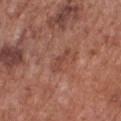notes: catalogued during a skin exam; not biopsied
location: the chest
illumination: white-light
image: total-body-photography crop, ~15 mm field of view
subject: male, roughly 75 years of age
size: ~3 mm (longest diameter)
TBP lesion metrics: a footprint of about 2.5 mm² and an outline eccentricity of about 0.95 (0 = round, 1 = elongated); a mean CIELAB color near L≈45 a*≈24 b*≈28; border irregularity of about 5.5 on a 0–10 scale, internal color variation of about 0 on a 0–10 scale, and a peripheral color-asymmetry measure near 0